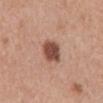Recorded during total-body skin imaging; not selected for excision or biopsy.
A male subject aged 68 to 72.
A 15 mm close-up tile from a total-body photography series done for melanoma screening.
The lesion is on the abdomen.
Captured under white-light illumination.